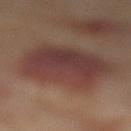Located on the left lower leg.
Approximately 7.5 mm at its widest.
This is a cross-polarized tile.
A 15 mm close-up extracted from a 3D total-body photography capture.
The total-body-photography lesion software estimated a footprint of about 36 mm² and a shape eccentricity near 0.5. And it measured a border-irregularity index near 2.5/10, a color-variation rating of about 5/10, and radial color variation of about 1.5. The analysis additionally found a lesion-detection confidence of about 100/100.
A female subject in their mid- to late 50s.